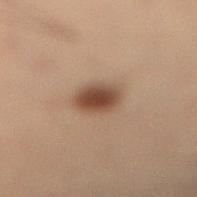- biopsy status — imaged on a skin check; not biopsied
- location — the right lower leg
- subject — male, aged 53–57
- tile lighting — cross-polarized illumination
- imaging modality — total-body-photography crop, ~15 mm field of view
- lesion diameter — ≈3 mm